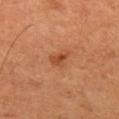Findings:
– notes: imaged on a skin check; not biopsied
– patient: male, approximately 50 years of age
– body site: the left thigh
– image source: ~15 mm tile from a whole-body skin photo